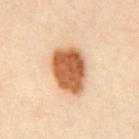Background: The patient is a male aged approximately 40. Measured at roughly 5.5 mm in maximum diameter. On the abdomen. A lesion tile, about 15 mm wide, cut from a 3D total-body photograph.